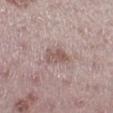{
  "biopsy_status": "not biopsied; imaged during a skin examination",
  "automated_metrics": {
    "area_mm2_approx": 5.0,
    "shape_asymmetry": 0.25,
    "border_irregularity_0_10": 3.0,
    "color_variation_0_10": 3.0,
    "peripheral_color_asymmetry": 1.0,
    "nevus_likeness_0_100": 10
  },
  "site": "right lower leg",
  "lighting": "white-light",
  "image": {
    "source": "total-body photography crop",
    "field_of_view_mm": 15
  },
  "patient": {
    "sex": "female",
    "age_approx": 45
  },
  "lesion_size": {
    "long_diameter_mm_approx": 3.0
  }
}